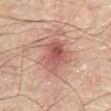notes: no biopsy performed (imaged during a skin exam)
tile lighting: cross-polarized illumination
acquisition: ~15 mm crop, total-body skin-cancer survey
site: the abdomen
patient: male, approximately 70 years of age
diameter: about 4 mm